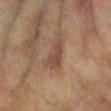Clinical impression:
Imaged during a routine full-body skin examination; the lesion was not biopsied and no histopathology is available.
Image and clinical context:
A close-up tile cropped from a whole-body skin photograph, about 15 mm across. A female patient, aged around 75. The lesion is on the left forearm.